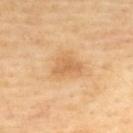{
  "biopsy_status": "not biopsied; imaged during a skin examination",
  "patient": {
    "sex": "female",
    "age_approx": 45
  },
  "image": {
    "source": "total-body photography crop",
    "field_of_view_mm": 15
  },
  "site": "upper back"
}